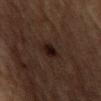biopsy status = total-body-photography surveillance lesion; no biopsy | tile lighting = cross-polarized illumination | acquisition = total-body-photography crop, ~15 mm field of view | lesion diameter = about 3 mm | anatomic site = the back | automated lesion analysis = an average lesion color of about L≈14 a*≈13 b*≈15 (CIELAB), about 8 CIELAB-L* units darker than the surrounding skin, and a normalized border contrast of about 11; an automated nevus-likeness rating near 100 out of 100 | subject = male, in their mid-80s or older.